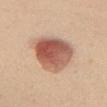Notes:
* workup · imaged on a skin check; not biopsied
* acquisition · total-body-photography crop, ~15 mm field of view
* site · the chest
* illumination · white-light illumination
* diameter · ≈6 mm
* patient · female, aged around 50